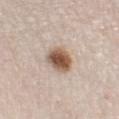Captured during whole-body skin photography for melanoma surveillance; the lesion was not biopsied. Captured under white-light illumination. The lesion is on the left lower leg. This image is a 15 mm lesion crop taken from a total-body photograph. The patient is a male aged 78 to 82.